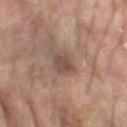Case summary:
– notes: total-body-photography surveillance lesion; no biopsy
– patient: female, aged around 75
– body site: the left thigh
– TBP lesion metrics: an outline eccentricity of about 0.75 (0 = round, 1 = elongated) and a symmetry-axis asymmetry near 0.25; border irregularity of about 2.5 on a 0–10 scale and radial color variation of about 1.5; an automated nevus-likeness rating near 0 out of 100 and lesion-presence confidence of about 100/100
– illumination: cross-polarized
– imaging modality: ~15 mm tile from a whole-body skin photo
– diameter: ~3 mm (longest diameter)